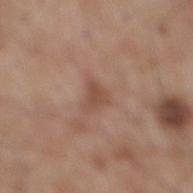biopsy_status: not biopsied; imaged during a skin examination
automated_metrics:
  eccentricity: 0.6
  shape_asymmetry: 0.45
  cielab_L: 49
  cielab_a: 20
  cielab_b: 28
  vs_skin_darker_L: 8.0
  vs_skin_contrast_norm: 6.5
  border_irregularity_0_10: 4.0
  color_variation_0_10: 1.5
  peripheral_color_asymmetry: 0.5
  nevus_likeness_0_100: 0
  lesion_detection_confidence_0_100: 100
lesion_size:
  long_diameter_mm_approx: 2.5
site: mid back
image:
  source: total-body photography crop
  field_of_view_mm: 15
patient:
  sex: male
  age_approx: 60
lighting: white-light